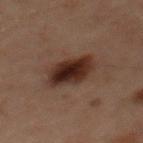Notes:
• biopsy status · total-body-photography surveillance lesion; no biopsy
• location · the back
• subject · male, roughly 60 years of age
• imaging modality · ~15 mm crop, total-body skin-cancer survey
• lesion size · ≈5.5 mm
• tile lighting · cross-polarized illumination
• automated metrics · a mean CIELAB color near L≈21 a*≈14 b*≈18, a lesion–skin lightness drop of about 10, and a lesion-to-skin contrast of about 12 (normalized; higher = more distinct); border irregularity of about 1.5 on a 0–10 scale and peripheral color asymmetry of about 1.5